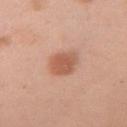Imaged during a routine full-body skin examination; the lesion was not biopsied and no histopathology is available. The tile uses white-light illumination. The lesion is on the left upper arm. The lesion's longest dimension is about 3.5 mm. The subject is a female in their 50s. This image is a 15 mm lesion crop taken from a total-body photograph.